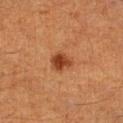- patient — male, aged around 60
- location — the left lower leg
- imaging modality — total-body-photography crop, ~15 mm field of view